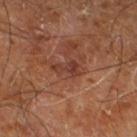follow-up — imaged on a skin check; not biopsied
lesion diameter — ≈3 mm
lighting — cross-polarized
location — the right leg
TBP lesion metrics — an area of roughly 3.5 mm², an eccentricity of roughly 0.85, and a shape-asymmetry score of about 0.65 (0 = symmetric); a border-irregularity rating of about 7.5/10, a color-variation rating of about 1.5/10, and peripheral color asymmetry of about 0.5; a classifier nevus-likeness of about 0/100 and a lesion-detection confidence of about 100/100
subject — male, approximately 60 years of age
imaging modality — 15 mm crop, total-body photography